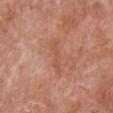<tbp_lesion>
  <biopsy_status>not biopsied; imaged during a skin examination</biopsy_status>
  <image>
    <source>total-body photography crop</source>
    <field_of_view_mm>15</field_of_view_mm>
  </image>
  <patient>
    <sex>female</sex>
    <age_approx>60</age_approx>
  </patient>
  <site>chest</site>
</tbp_lesion>